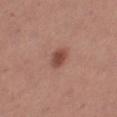Q: Is there a histopathology result?
A: no biopsy performed (imaged during a skin exam)
Q: How was the tile lit?
A: white-light
Q: Patient demographics?
A: female, approximately 40 years of age
Q: Where on the body is the lesion?
A: the right thigh
Q: How was this image acquired?
A: ~15 mm tile from a whole-body skin photo